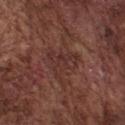Automated tile analysis of the lesion measured a lesion area of about 8.5 mm², an eccentricity of roughly 0.45, and two-axis asymmetry of about 0.6. The software also gave a lesion color around L≈32 a*≈21 b*≈22 in CIELAB, a lesion–skin lightness drop of about 6, and a normalized border contrast of about 5.5. It also reported an automated nevus-likeness rating near 0 out of 100 and a detector confidence of about 90 out of 100 that the crop contains a lesion. A male patient roughly 75 years of age. Captured under white-light illumination. On the front of the torso. A lesion tile, about 15 mm wide, cut from a 3D total-body photograph. Measured at roughly 4 mm in maximum diameter.